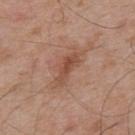follow-up: total-body-photography surveillance lesion; no biopsy | anatomic site: the mid back | size: about 4.5 mm | image: ~15 mm tile from a whole-body skin photo | tile lighting: white-light illumination | subject: male, in their mid- to late 50s.